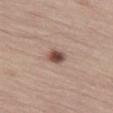Q: What are the patient's age and sex?
A: male, in their mid- to late 60s
Q: What is the anatomic site?
A: the leg
Q: What kind of image is this?
A: total-body-photography crop, ~15 mm field of view